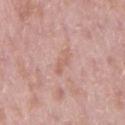workup = no biopsy performed (imaged during a skin exam) | image source = ~15 mm tile from a whole-body skin photo | site = the right upper arm | illumination = white-light | lesion diameter = ≈3.5 mm | patient = male, aged approximately 50.